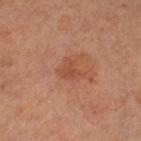Assessment:
The lesion was tiled from a total-body skin photograph and was not biopsied.
Background:
This image is a 15 mm lesion crop taken from a total-body photograph. A female patient aged 63–67. This is a cross-polarized tile. The lesion is on the left thigh. The recorded lesion diameter is about 3 mm.